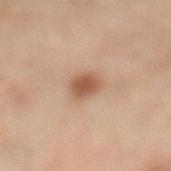Q: Is there a histopathology result?
A: catalogued during a skin exam; not biopsied
Q: Automated lesion metrics?
A: a mean CIELAB color near L≈56 a*≈20 b*≈32 and about 12 CIELAB-L* units darker than the surrounding skin
Q: What are the patient's age and sex?
A: male, aged around 55
Q: What is the imaging modality?
A: ~15 mm tile from a whole-body skin photo
Q: Lesion location?
A: the left lower leg
Q: Illumination type?
A: cross-polarized